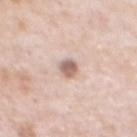No biopsy was performed on this lesion — it was imaged during a full skin examination and was not determined to be concerning. The total-body-photography lesion software estimated a lesion-to-skin contrast of about 8.5 (normalized; higher = more distinct). Longest diameter approximately 2.5 mm. On the chest. A male subject, aged around 80. A 15 mm close-up extracted from a 3D total-body photography capture. This is a white-light tile.